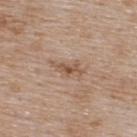Q: Is there a histopathology result?
A: catalogued during a skin exam; not biopsied
Q: What is the imaging modality?
A: ~15 mm tile from a whole-body skin photo
Q: Lesion location?
A: the upper back
Q: What are the patient's age and sex?
A: male, aged 73–77
Q: What did automated image analysis measure?
A: an average lesion color of about L≈53 a*≈18 b*≈30 (CIELAB), about 10 CIELAB-L* units darker than the surrounding skin, and a normalized lesion–skin contrast near 7; a border-irregularity index near 6/10 and peripheral color asymmetry of about 0.5